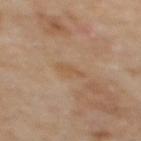Assessment:
Captured during whole-body skin photography for melanoma surveillance; the lesion was not biopsied.
Context:
Captured under cross-polarized illumination. A roughly 15 mm field-of-view crop from a total-body skin photograph. An algorithmic analysis of the crop reported an area of roughly 3 mm², a shape eccentricity near 0.85, and a symmetry-axis asymmetry near 0.3. And it measured a classifier nevus-likeness of about 0/100 and a lesion-detection confidence of about 100/100. From the upper back. A female patient, aged approximately 60. Measured at roughly 3 mm in maximum diameter.